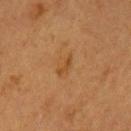Clinical impression: The lesion was photographed on a routine skin check and not biopsied; there is no pathology result. Image and clinical context: Automated tile analysis of the lesion measured a shape eccentricity near 0.9 and two-axis asymmetry of about 0.55. The analysis additionally found a border-irregularity index near 5.5/10, internal color variation of about 0 on a 0–10 scale, and radial color variation of about 0. The software also gave a classifier nevus-likeness of about 5/100 and a detector confidence of about 100 out of 100 that the crop contains a lesion. A 15 mm close-up extracted from a 3D total-body photography capture. A female subject approximately 55 years of age. From the left upper arm.